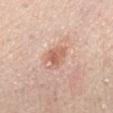| key | value |
|---|---|
| follow-up | catalogued during a skin exam; not biopsied |
| subject | male, in their 50s |
| image-analysis metrics | two-axis asymmetry of about 0.4; a lesion color around L≈62 a*≈23 b*≈30 in CIELAB, about 10 CIELAB-L* units darker than the surrounding skin, and a normalized lesion–skin contrast near 6.5; lesion-presence confidence of about 100/100 |
| size | ≈3 mm |
| image source | total-body-photography crop, ~15 mm field of view |
| illumination | white-light |
| anatomic site | the mid back |